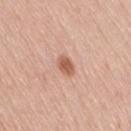Part of a total-body skin-imaging series; this lesion was reviewed on a skin check and was not flagged for biopsy. Located on the lower back. Longest diameter approximately 2.5 mm. The patient is a female aged around 70. A roughly 15 mm field-of-view crop from a total-body skin photograph. An algorithmic analysis of the crop reported a shape eccentricity near 0.7 and a symmetry-axis asymmetry near 0.15. It also reported border irregularity of about 1.5 on a 0–10 scale, a color-variation rating of about 2.5/10, and a peripheral color-asymmetry measure near 0.5. The software also gave an automated nevus-likeness rating near 95 out of 100 and a lesion-detection confidence of about 100/100. Imaged with white-light lighting.